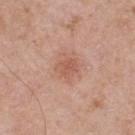Part of a total-body skin-imaging series; this lesion was reviewed on a skin check and was not flagged for biopsy. A roughly 15 mm field-of-view crop from a total-body skin photograph. This is a white-light tile. Automated tile analysis of the lesion measured a lesion area of about 4.5 mm², a shape eccentricity near 0.5, and two-axis asymmetry of about 0.2. The analysis additionally found a border-irregularity index near 2/10, a within-lesion color-variation index near 2.5/10, and radial color variation of about 1. Longest diameter approximately 2.5 mm. A male patient aged 53–57. The lesion is on the back.